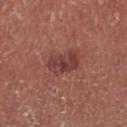The lesion was photographed on a routine skin check and not biopsied; there is no pathology result. The lesion is on the right lower leg. Longest diameter approximately 4 mm. The subject is a male aged 68 to 72. A roughly 15 mm field-of-view crop from a total-body skin photograph. Captured under white-light illumination.